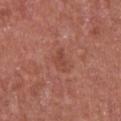| field | value |
|---|---|
| workup | total-body-photography surveillance lesion; no biopsy |
| image source | 15 mm crop, total-body photography |
| patient | male, aged 63–67 |
| site | the chest |
| size | ≈3 mm |
| lighting | white-light |